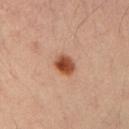biopsy_status: not biopsied; imaged during a skin examination